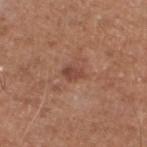Imaged during a routine full-body skin examination; the lesion was not biopsied and no histopathology is available.
Longest diameter approximately 2.5 mm.
Located on the left lower leg.
The total-body-photography lesion software estimated an area of roughly 3.5 mm², a shape eccentricity near 0.75, and two-axis asymmetry of about 0.3. The software also gave a border-irregularity index near 3/10, a color-variation rating of about 2.5/10, and a peripheral color-asymmetry measure near 1.
Cropped from a whole-body photographic skin survey; the tile spans about 15 mm.
The subject is a male in their mid- to late 70s.
The tile uses white-light illumination.